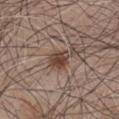A male patient roughly 65 years of age. About 3 mm across. This is a white-light tile. Cropped from a total-body skin-imaging series; the visible field is about 15 mm. On the chest.